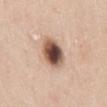This lesion was catalogued during total-body skin photography and was not selected for biopsy. A male patient, aged 38–42. This image is a 15 mm lesion crop taken from a total-body photograph. The tile uses white-light illumination. Measured at roughly 4 mm in maximum diameter. Located on the back.